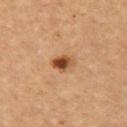site = the arm; illumination = cross-polarized illumination; subject = female, roughly 45 years of age; acquisition = 15 mm crop, total-body photography; size = ~2.5 mm (longest diameter).